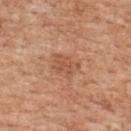A male subject, aged approximately 60.
A 15 mm close-up extracted from a 3D total-body photography capture.
On the upper back.